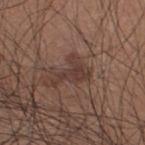{"biopsy_status": "not biopsied; imaged during a skin examination", "image": {"source": "total-body photography crop", "field_of_view_mm": 15}, "patient": {"sex": "male", "age_approx": 35}, "lighting": "white-light", "lesion_size": {"long_diameter_mm_approx": 4.5}, "site": "upper back"}